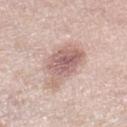Assessment: This lesion was catalogued during total-body skin photography and was not selected for biopsy. Background: Automated tile analysis of the lesion measured a footprint of about 16 mm², a shape eccentricity near 0.65, and a shape-asymmetry score of about 0.3 (0 = symmetric). And it measured a lesion color around L≈62 a*≈19 b*≈22 in CIELAB and a lesion-to-skin contrast of about 7.5 (normalized; higher = more distinct). The software also gave a classifier nevus-likeness of about 25/100. A female patient, roughly 40 years of age. The recorded lesion diameter is about 5.5 mm. The lesion is located on the right lower leg. A close-up tile cropped from a whole-body skin photograph, about 15 mm across. The tile uses white-light illumination.